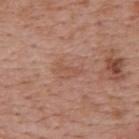Q: Is there a histopathology result?
A: total-body-photography surveillance lesion; no biopsy
Q: What are the patient's age and sex?
A: female, aged around 40
Q: What is the anatomic site?
A: the upper back
Q: What kind of image is this?
A: ~15 mm tile from a whole-body skin photo
Q: How large is the lesion?
A: about 3 mm
Q: What lighting was used for the tile?
A: white-light